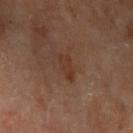Impression:
This lesion was catalogued during total-body skin photography and was not selected for biopsy.
Clinical summary:
A close-up tile cropped from a whole-body skin photograph, about 15 mm across. Longest diameter approximately 3 mm. The subject is a male in their mid- to late 80s or older. From the left upper arm. Captured under cross-polarized illumination.